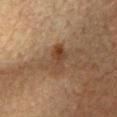Clinical impression:
No biopsy was performed on this lesion — it was imaged during a full skin examination and was not determined to be concerning.
Context:
Automated tile analysis of the lesion measured a lesion color around L≈38 a*≈16 b*≈29 in CIELAB, about 7 CIELAB-L* units darker than the surrounding skin, and a lesion-to-skin contrast of about 7 (normalized; higher = more distinct). It also reported a classifier nevus-likeness of about 0/100. A lesion tile, about 15 mm wide, cut from a 3D total-body photograph. The tile uses cross-polarized illumination. The lesion is located on the chest. A male subject in their mid-60s. Longest diameter approximately 4 mm.